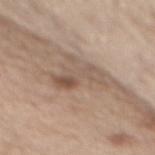* notes — total-body-photography surveillance lesion; no biopsy
* patient — male, approximately 70 years of age
* illumination — white-light
* lesion size — about 5 mm
* body site — the back
* acquisition — ~15 mm tile from a whole-body skin photo